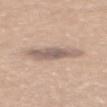<lesion>
<biopsy_status>not biopsied; imaged during a skin examination</biopsy_status>
<patient>
  <sex>female</sex>
  <age_approx>55</age_approx>
</patient>
<lighting>white-light</lighting>
<lesion_size>
  <long_diameter_mm_approx>5.0</long_diameter_mm_approx>
</lesion_size>
<image>
  <source>total-body photography crop</source>
  <field_of_view_mm>15</field_of_view_mm>
</image>
<site>upper back</site>
</lesion>